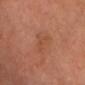The lesion is on the head or neck. A 15 mm close-up extracted from a 3D total-body photography capture. The lesion's longest dimension is about 5 mm. This is a cross-polarized tile.